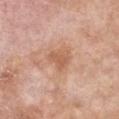lesion size = ~3 mm (longest diameter) | imaging modality = ~15 mm tile from a whole-body skin photo | location = the chest | subject = female, roughly 75 years of age.